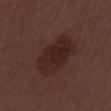Image and clinical context: The lesion is located on the mid back. The lesion's longest dimension is about 6 mm. A 15 mm close-up tile from a total-body photography series done for melanoma screening. This is a white-light tile. A male patient in their 30s.